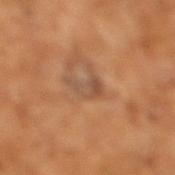Impression:
Recorded during total-body skin imaging; not selected for excision or biopsy.
Context:
Imaged with cross-polarized lighting. Automated image analysis of the tile measured roughly 7 lightness units darker than nearby skin and a lesion-to-skin contrast of about 6.5 (normalized; higher = more distinct). The analysis additionally found a border-irregularity index near 5.5/10. And it measured an automated nevus-likeness rating near 0 out of 100. Longest diameter approximately 3.5 mm. A male subject approximately 65 years of age. A lesion tile, about 15 mm wide, cut from a 3D total-body photograph.